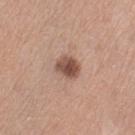Impression:
Recorded during total-body skin imaging; not selected for excision or biopsy.